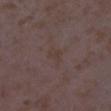| feature | finding |
|---|---|
| biopsy status | catalogued during a skin exam; not biopsied |
| tile lighting | white-light illumination |
| TBP lesion metrics | a nevus-likeness score of about 0/100 and lesion-presence confidence of about 100/100 |
| image | 15 mm crop, total-body photography |
| size | ~3 mm (longest diameter) |
| patient | female, aged 33–37 |
| location | the right lower leg |